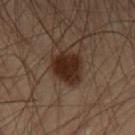Assessment: Imaged during a routine full-body skin examination; the lesion was not biopsied and no histopathology is available. Background: A close-up tile cropped from a whole-body skin photograph, about 15 mm across. Automated tile analysis of the lesion measured a footprint of about 11 mm² and two-axis asymmetry of about 0.2. It also reported an average lesion color of about L≈20 a*≈14 b*≈20 (CIELAB), about 11 CIELAB-L* units darker than the surrounding skin, and a normalized lesion–skin contrast near 12.5. The analysis additionally found lesion-presence confidence of about 100/100. The lesion is on the chest. This is a cross-polarized tile. Approximately 4.5 mm at its widest. A male subject roughly 55 years of age.